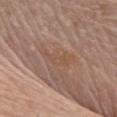lighting: white-light illumination | lesion size: about 4.5 mm | acquisition: 15 mm crop, total-body photography | anatomic site: the front of the torso | subject: female, about 80 years old.